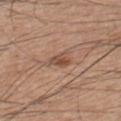  site: front of the torso
  patient:
    sex: male
    age_approx: 60
  lesion_size:
    long_diameter_mm_approx: 2.5
  image:
    source: total-body photography crop
    field_of_view_mm: 15
  automated_metrics:
    vs_skin_darker_L: 10.0
    vs_skin_contrast_norm: 7.0
    nevus_likeness_0_100: 25
    lesion_detection_confidence_0_100: 100
  lighting: white-light